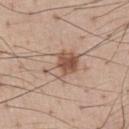- notes: no biopsy performed (imaged during a skin exam)
- lighting: white-light
- location: the chest
- image source: 15 mm crop, total-body photography
- diameter: ≈5 mm
- subject: male, aged 53–57